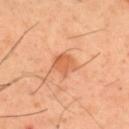Imaged during a routine full-body skin examination; the lesion was not biopsied and no histopathology is available.
Cropped from a whole-body photographic skin survey; the tile spans about 15 mm.
Located on the mid back.
A male patient aged approximately 40.
The tile uses cross-polarized illumination.
Approximately 2.5 mm at its widest.